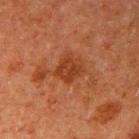Assessment: Imaged during a routine full-body skin examination; the lesion was not biopsied and no histopathology is available. Clinical summary: Automated tile analysis of the lesion measured a footprint of about 7.5 mm². It also reported a mean CIELAB color near L≈31 a*≈24 b*≈30 and a normalized lesion–skin contrast near 7. The software also gave border irregularity of about 2.5 on a 0–10 scale and peripheral color asymmetry of about 1. The tile uses cross-polarized illumination. The lesion's longest dimension is about 3 mm. On the right upper arm. A close-up tile cropped from a whole-body skin photograph, about 15 mm across. A male subject aged approximately 60.